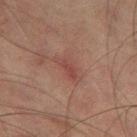- workup — imaged on a skin check; not biopsied
- size — ≈3 mm
- anatomic site — the leg
- subject — male, aged around 75
- illumination — cross-polarized illumination
- image source — total-body-photography crop, ~15 mm field of view